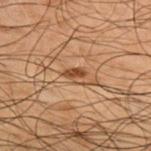<record>
  <biopsy_status>not biopsied; imaged during a skin examination</biopsy_status>
  <automated_metrics>
    <area_mm2_approx>3.5</area_mm2_approx>
    <eccentricity>0.75</eccentricity>
    <shape_asymmetry>0.35</shape_asymmetry>
    <cielab_L>37</cielab_L>
    <cielab_a>17</cielab_a>
    <cielab_b>28</cielab_b>
    <vs_skin_darker_L>9.0</vs_skin_darker_L>
    <nevus_likeness_0_100>80</nevus_likeness_0_100>
    <lesion_detection_confidence_0_100>95</lesion_detection_confidence_0_100>
  </automated_metrics>
  <patient>
    <sex>male</sex>
    <age_approx>50</age_approx>
  </patient>
  <image>
    <source>total-body photography crop</source>
    <field_of_view_mm>15</field_of_view_mm>
  </image>
  <lighting>cross-polarized</lighting>
  <site>upper back</site>
  <lesion_size>
    <long_diameter_mm_approx>2.5</long_diameter_mm_approx>
  </lesion_size>
</record>